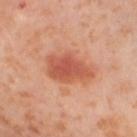biopsy status: no biopsy performed (imaged during a skin exam)
automated lesion analysis: a lesion color around L≈56 a*≈30 b*≈34 in CIELAB and a lesion–skin lightness drop of about 11; a classifier nevus-likeness of about 100/100
site: the left thigh
subject: female, in their mid- to late 50s
image source: total-body-photography crop, ~15 mm field of view